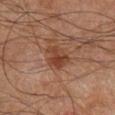notes: imaged on a skin check; not biopsied
imaging modality: 15 mm crop, total-body photography
body site: the right lower leg
lighting: cross-polarized
lesion diameter: ~3.5 mm (longest diameter)
image-analysis metrics: an automated nevus-likeness rating near 50 out of 100 and a detector confidence of about 100 out of 100 that the crop contains a lesion
subject: male, aged around 65